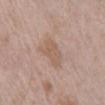location: the abdomen
TBP lesion metrics: a classifier nevus-likeness of about 0/100
imaging modality: 15 mm crop, total-body photography
patient: male, aged around 60
size: about 4 mm